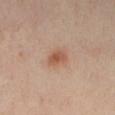Background: Approximately 2.5 mm at its widest. Located on the right leg. A lesion tile, about 15 mm wide, cut from a 3D total-body photograph. This is a cross-polarized tile. The subject is a female about 40 years old. Automated tile analysis of the lesion measured a footprint of about 4 mm², an outline eccentricity of about 0.75 (0 = round, 1 = elongated), and a symmetry-axis asymmetry near 0.25. It also reported a border-irregularity rating of about 2/10, internal color variation of about 4 on a 0–10 scale, and a peripheral color-asymmetry measure near 1.5. And it measured a lesion-detection confidence of about 100/100.